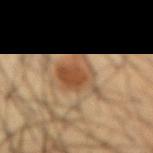| field | value |
|---|---|
| lesion size | about 5 mm |
| imaging modality | ~15 mm crop, total-body skin-cancer survey |
| patient | male, in their mid- to late 40s |
| image-analysis metrics | an area of roughly 14 mm², an eccentricity of roughly 0.4, and two-axis asymmetry of about 0.35; internal color variation of about 5 on a 0–10 scale and radial color variation of about 1.5; a classifier nevus-likeness of about 95/100 and a detector confidence of about 100 out of 100 that the crop contains a lesion |
| anatomic site | the abdomen |
| illumination | cross-polarized |